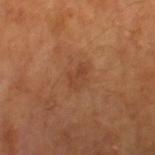The lesion was photographed on a routine skin check and not biopsied; there is no pathology result.
Cropped from a whole-body photographic skin survey; the tile spans about 15 mm.
A male subject, roughly 65 years of age.